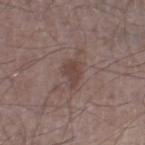Q: Was a biopsy performed?
A: catalogued during a skin exam; not biopsied
Q: How was the tile lit?
A: white-light
Q: Lesion size?
A: ~3.5 mm (longest diameter)
Q: What is the imaging modality?
A: ~15 mm tile from a whole-body skin photo
Q: Where on the body is the lesion?
A: the left thigh
Q: What did automated image analysis measure?
A: a shape eccentricity near 0.85 and a shape-asymmetry score of about 0.3 (0 = symmetric); a border-irregularity index near 3.5/10 and peripheral color asymmetry of about 0.5
Q: Who is the patient?
A: male, aged 63–67